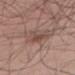No biopsy was performed on this lesion — it was imaged during a full skin examination and was not determined to be concerning. A 15 mm crop from a total-body photograph taken for skin-cancer surveillance. A male subject aged around 60. On the leg. This is a white-light tile.